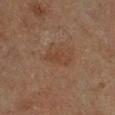<tbp_lesion>
  <biopsy_status>not biopsied; imaged during a skin examination</biopsy_status>
  <lesion_size>
    <long_diameter_mm_approx>3.0</long_diameter_mm_approx>
  </lesion_size>
  <patient>
    <sex>male</sex>
    <age_approx>85+</age_approx>
  </patient>
  <lighting>cross-polarized</lighting>
  <image>
    <source>total-body photography crop</source>
    <field_of_view_mm>15</field_of_view_mm>
  </image>
  <site>left lower leg</site>
</tbp_lesion>